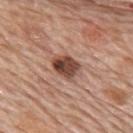No biopsy was performed on this lesion — it was imaged during a full skin examination and was not determined to be concerning. Captured under white-light illumination. Measured at roughly 3.5 mm in maximum diameter. Located on the upper back. A lesion tile, about 15 mm wide, cut from a 3D total-body photograph. The lesion-visualizer software estimated about 16 CIELAB-L* units darker than the surrounding skin and a lesion-to-skin contrast of about 11 (normalized; higher = more distinct). And it measured an automated nevus-likeness rating near 95 out of 100 and lesion-presence confidence of about 100/100. A female patient roughly 65 years of age.